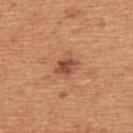Assessment:
The lesion was tiled from a total-body skin photograph and was not biopsied.
Background:
Imaged with white-light lighting. The recorded lesion diameter is about 3 mm. The total-body-photography lesion software estimated about 12 CIELAB-L* units darker than the surrounding skin and a normalized lesion–skin contrast near 8.5. The software also gave a classifier nevus-likeness of about 50/100. This image is a 15 mm lesion crop taken from a total-body photograph. The subject is a male approximately 55 years of age. The lesion is on the upper back.